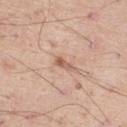Notes:
• notes · total-body-photography surveillance lesion; no biopsy
• size · ≈3 mm
• tile lighting · white-light
• TBP lesion metrics · a peripheral color-asymmetry measure near 0; a detector confidence of about 100 out of 100 that the crop contains a lesion
• subject · male, aged 58–62
• image · total-body-photography crop, ~15 mm field of view
• location · the right thigh